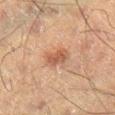notes = total-body-photography surveillance lesion; no biopsy